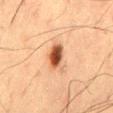Impression:
Imaged during a routine full-body skin examination; the lesion was not biopsied and no histopathology is available.
Context:
The tile uses cross-polarized illumination. A close-up tile cropped from a whole-body skin photograph, about 15 mm across. A male patient aged 58 to 62. Longest diameter approximately 3.5 mm. The lesion is on the mid back.